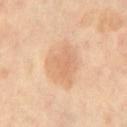Imaged during a routine full-body skin examination; the lesion was not biopsied and no histopathology is available.